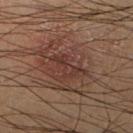A male patient roughly 40 years of age.
On the leg.
A region of skin cropped from a whole-body photographic capture, roughly 15 mm wide.
Captured under cross-polarized illumination.
The recorded lesion diameter is about 3 mm.
The total-body-photography lesion software estimated a footprint of about 3.5 mm², a shape eccentricity near 0.9, and two-axis asymmetry of about 0.4. The software also gave a border-irregularity rating of about 5/10.